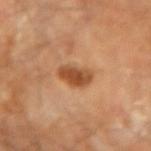biopsy status: no biopsy performed (imaged during a skin exam)
lighting: cross-polarized illumination
image: total-body-photography crop, ~15 mm field of view
subject: male, aged 83 or older
lesion diameter: about 4 mm
location: the left forearm
image-analysis metrics: a lesion area of about 6.5 mm², an outline eccentricity of about 0.8 (0 = round, 1 = elongated), and a symmetry-axis asymmetry near 0.3; a classifier nevus-likeness of about 95/100 and a detector confidence of about 100 out of 100 that the crop contains a lesion